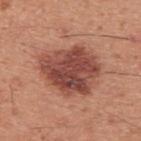<tbp_lesion>
<biopsy_status>not biopsied; imaged during a skin examination</biopsy_status>
<site>upper back</site>
<image>
  <source>total-body photography crop</source>
  <field_of_view_mm>15</field_of_view_mm>
</image>
<lesion_size>
  <long_diameter_mm_approx>7.0</long_diameter_mm_approx>
</lesion_size>
<patient>
  <sex>male</sex>
  <age_approx>45</age_approx>
</patient>
<automated_metrics>
  <area_mm2_approx>27.0</area_mm2_approx>
  <cielab_L>46</cielab_L>
  <cielab_a>26</cielab_a>
  <cielab_b>27</cielab_b>
  <vs_skin_darker_L>14.0</vs_skin_darker_L>
  <vs_skin_contrast_norm>10.0</vs_skin_contrast_norm>
  <border_irregularity_0_10>2.5</border_irregularity_0_10>
  <color_variation_0_10>5.5</color_variation_0_10>
  <peripheral_color_asymmetry>1.5</peripheral_color_asymmetry>
</automated_metrics>
<lighting>white-light</lighting>
</tbp_lesion>